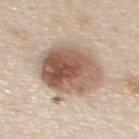biopsy status = imaged on a skin check; not biopsied
lesion diameter = about 8 mm
patient = female, aged approximately 45
image = ~15 mm tile from a whole-body skin photo
location = the back
lighting = white-light